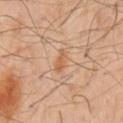biopsy_status: not biopsied; imaged during a skin examination
automated_metrics:
  area_mm2_approx: 3.5
  eccentricity: 0.85
  shape_asymmetry: 0.35
  border_irregularity_0_10: 4.0
  color_variation_0_10: 1.5
  peripheral_color_asymmetry: 0.5
patient:
  sex: male
  age_approx: 60
lesion_size:
  long_diameter_mm_approx: 3.0
lighting: cross-polarized
image:
  source: total-body photography crop
  field_of_view_mm: 15
site: chest